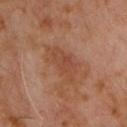location: the front of the torso
tile lighting: cross-polarized illumination
subject: male, approximately 60 years of age
TBP lesion metrics: an average lesion color of about L≈43 a*≈22 b*≈29 (CIELAB), a lesion–skin lightness drop of about 6, and a lesion-to-skin contrast of about 5.5 (normalized; higher = more distinct)
diameter: ≈5 mm
image: ~15 mm crop, total-body skin-cancer survey